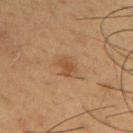Part of a total-body skin-imaging series; this lesion was reviewed on a skin check and was not flagged for biopsy.